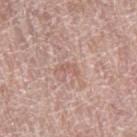Notes:
• workup — total-body-photography surveillance lesion; no biopsy
• lighting — white-light
• location — the leg
• imaging modality — total-body-photography crop, ~15 mm field of view
• TBP lesion metrics — internal color variation of about 0 on a 0–10 scale and a peripheral color-asymmetry measure near 0
• patient — female, in their 60s
• lesion diameter — about 2.5 mm